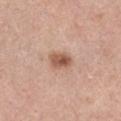Q: Is there a histopathology result?
A: catalogued during a skin exam; not biopsied